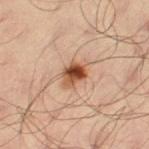Clinical impression:
Imaged during a routine full-body skin examination; the lesion was not biopsied and no histopathology is available.
Context:
Imaged with cross-polarized lighting. A 15 mm close-up extracted from a 3D total-body photography capture. The total-body-photography lesion software estimated border irregularity of about 2.5 on a 0–10 scale, a color-variation rating of about 9.5/10, and radial color variation of about 3.5. The lesion is located on the leg. The subject is a male roughly 35 years of age. The lesion's longest dimension is about 3 mm.